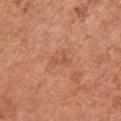| feature | finding |
|---|---|
| workup | imaged on a skin check; not biopsied |
| subject | female, aged 63 to 67 |
| lighting | white-light illumination |
| imaging modality | ~15 mm tile from a whole-body skin photo |
| site | the arm |